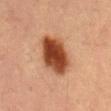Q: Was a biopsy performed?
A: imaged on a skin check; not biopsied
Q: Where on the body is the lesion?
A: the abdomen
Q: What is the lesion's diameter?
A: about 6 mm
Q: What are the patient's age and sex?
A: male, roughly 55 years of age
Q: What is the imaging modality?
A: ~15 mm tile from a whole-body skin photo